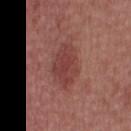Clinical impression:
The lesion was tiled from a total-body skin photograph and was not biopsied.
Context:
The total-body-photography lesion software estimated an outline eccentricity of about 0.8 (0 = round, 1 = elongated) and a shape-asymmetry score of about 0.15 (0 = symmetric). The analysis additionally found a normalized border contrast of about 7. The software also gave a nevus-likeness score of about 45/100. Imaged with white-light lighting. The subject is a male in their 30s. The lesion's longest dimension is about 5.5 mm. A close-up tile cropped from a whole-body skin photograph, about 15 mm across.